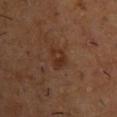imaging modality: ~15 mm crop, total-body skin-cancer survey
location: the upper back
subject: male, aged around 55